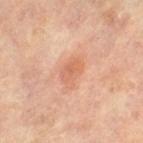workup — imaged on a skin check; not biopsied
imaging modality — ~15 mm crop, total-body skin-cancer survey
location — the right leg
TBP lesion metrics — an outline eccentricity of about 0.75 (0 = round, 1 = elongated) and a shape-asymmetry score of about 0.3 (0 = symmetric)
subject — female, aged around 65
illumination — cross-polarized
lesion diameter — about 3.5 mm